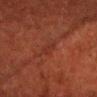Q: Was a biopsy performed?
A: catalogued during a skin exam; not biopsied
Q: What is the imaging modality?
A: total-body-photography crop, ~15 mm field of view
Q: What is the anatomic site?
A: the head or neck
Q: Patient demographics?
A: male, about 50 years old